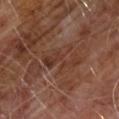| field | value |
|---|---|
| biopsy status | imaged on a skin check; not biopsied |
| image source | total-body-photography crop, ~15 mm field of view |
| automated lesion analysis | a lesion area of about 9 mm² and a symmetry-axis asymmetry near 0.45; a mean CIELAB color near L≈34 a*≈20 b*≈25 and roughly 6 lightness units darker than nearby skin; a color-variation rating of about 3.5/10 and peripheral color asymmetry of about 1; a nevus-likeness score of about 0/100 and lesion-presence confidence of about 60/100 |
| lesion size | about 5 mm |
| location | the front of the torso |
| patient | male, about 60 years old |